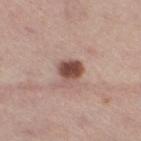{"biopsy_status": "not biopsied; imaged during a skin examination", "lesion_size": {"long_diameter_mm_approx": 3.0}, "lighting": "white-light", "patient": {"sex": "male", "age_approx": 75}, "site": "right thigh", "image": {"source": "total-body photography crop", "field_of_view_mm": 15}}